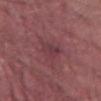Assessment: Imaged during a routine full-body skin examination; the lesion was not biopsied and no histopathology is available. Acquisition and patient details: A male patient, aged approximately 40. About 3.5 mm across. A region of skin cropped from a whole-body photographic capture, roughly 15 mm wide. On the abdomen.